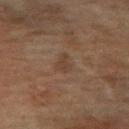The lesion was tiled from a total-body skin photograph and was not biopsied. A 15 mm close-up extracted from a 3D total-body photography capture. A female subject, approximately 55 years of age. The lesion's longest dimension is about 2.5 mm. Captured under cross-polarized illumination. The lesion is located on the right forearm.